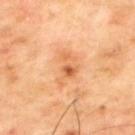Findings:
• subject: male, in their mid-60s
• acquisition: ~15 mm crop, total-body skin-cancer survey
• anatomic site: the back